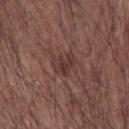Captured during whole-body skin photography for melanoma surveillance; the lesion was not biopsied. On the right forearm. The lesion-visualizer software estimated an eccentricity of roughly 0.55 and a symmetry-axis asymmetry near 0.35. It also reported a lesion color around L≈36 a*≈19 b*≈19 in CIELAB and a normalized border contrast of about 6.5. The software also gave a border-irregularity index near 4/10, internal color variation of about 3 on a 0–10 scale, and radial color variation of about 1. It also reported an automated nevus-likeness rating near 0 out of 100 and lesion-presence confidence of about 95/100. A 15 mm crop from a total-body photograph taken for skin-cancer surveillance. The lesion's longest dimension is about 3 mm. The subject is a male about 65 years old.